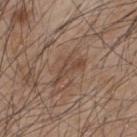This lesion was catalogued during total-body skin photography and was not selected for biopsy.
Measured at roughly 4.5 mm in maximum diameter.
A close-up tile cropped from a whole-body skin photograph, about 15 mm across.
This is a white-light tile.
Located on the upper back.
A male subject, aged approximately 45.
The lesion-visualizer software estimated an area of roughly 5 mm², an eccentricity of roughly 0.95, and two-axis asymmetry of about 0.6. The software also gave an average lesion color of about L≈45 a*≈17 b*≈27 (CIELAB) and a normalized border contrast of about 6.